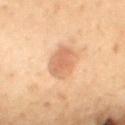biopsy status: catalogued during a skin exam; not biopsied | acquisition: 15 mm crop, total-body photography | automated metrics: a footprint of about 11 mm² and an outline eccentricity of about 0.75 (0 = round, 1 = elongated); an average lesion color of about L≈54 a*≈19 b*≈30 (CIELAB) | site: the mid back | lighting: cross-polarized | patient: male, aged approximately 55.